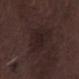No biopsy was performed on this lesion — it was imaged during a full skin examination and was not determined to be concerning.
A male patient, aged 73 to 77.
This image is a 15 mm lesion crop taken from a total-body photograph.
Located on the leg.